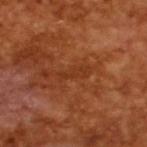A male subject in their mid-60s. Cropped from a whole-body photographic skin survey; the tile spans about 15 mm. Approximately 4 mm at its widest.